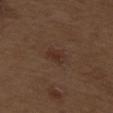notes: imaged on a skin check; not biopsied
location: the upper back
patient: male, aged 68 to 72
image-analysis metrics: a border-irregularity index near 2.5/10, a within-lesion color-variation index near 2.5/10, and peripheral color asymmetry of about 0.5; a lesion-detection confidence of about 100/100
lesion diameter: ~3 mm (longest diameter)
image: total-body-photography crop, ~15 mm field of view
illumination: white-light